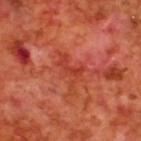Clinical impression:
The lesion was photographed on a routine skin check and not biopsied; there is no pathology result.
Background:
An algorithmic analysis of the crop reported an area of roughly 6.5 mm², an eccentricity of roughly 0.85, and a shape-asymmetry score of about 0.6 (0 = symmetric). It also reported about 7 CIELAB-L* units darker than the surrounding skin and a normalized border contrast of about 6. The analysis additionally found an automated nevus-likeness rating near 0 out of 100 and a lesion-detection confidence of about 95/100. Imaged with cross-polarized lighting. A 15 mm crop from a total-body photograph taken for skin-cancer surveillance. A male subject, in their 70s. On the upper back.